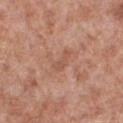Notes:
- notes · no biopsy performed (imaged during a skin exam)
- TBP lesion metrics · an eccentricity of roughly 0.85; a border-irregularity rating of about 5/10 and internal color variation of about 0.5 on a 0–10 scale
- lesion size · ~2.5 mm (longest diameter)
- anatomic site · the left lower leg
- patient · male, roughly 55 years of age
- imaging modality · 15 mm crop, total-body photography
- illumination · white-light illumination